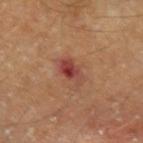Q: Is there a histopathology result?
A: imaged on a skin check; not biopsied
Q: What did automated image analysis measure?
A: a shape eccentricity near 0.8 and a shape-asymmetry score of about 0.25 (0 = symmetric); border irregularity of about 3 on a 0–10 scale, a color-variation rating of about 7.5/10, and a peripheral color-asymmetry measure near 2.5
Q: Patient demographics?
A: male, aged around 70
Q: What is the imaging modality?
A: total-body-photography crop, ~15 mm field of view
Q: Lesion location?
A: the left forearm
Q: Illumination type?
A: cross-polarized